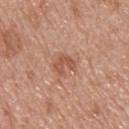Notes:
• diameter: ~2.5 mm (longest diameter)
• image: 15 mm crop, total-body photography
• lighting: white-light
• automated metrics: border irregularity of about 5.5 on a 0–10 scale, a color-variation rating of about 0/10, and peripheral color asymmetry of about 0; a lesion-detection confidence of about 100/100
• patient: male, in their mid- to late 50s
• location: the upper back